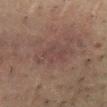follow-up: total-body-photography surveillance lesion; no biopsy
imaging modality: 15 mm crop, total-body photography
patient: male, in their 50s
size: ~5 mm (longest diameter)
anatomic site: the abdomen
illumination: cross-polarized
automated lesion analysis: a lesion area of about 12 mm² and two-axis asymmetry of about 0.25; a mean CIELAB color near L≈32 a*≈13 b*≈17, roughly 4 lightness units darker than nearby skin, and a normalized lesion–skin contrast near 4.5; border irregularity of about 4 on a 0–10 scale, internal color variation of about 2.5 on a 0–10 scale, and a peripheral color-asymmetry measure near 1; a nevus-likeness score of about 0/100 and a detector confidence of about 100 out of 100 that the crop contains a lesion